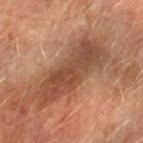Q: Is there a histopathology result?
A: catalogued during a skin exam; not biopsied
Q: Lesion location?
A: the left forearm
Q: What are the patient's age and sex?
A: male, roughly 65 years of age
Q: What kind of image is this?
A: total-body-photography crop, ~15 mm field of view